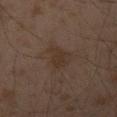The lesion was photographed on a routine skin check and not biopsied; there is no pathology result. The tile uses cross-polarized illumination. Cropped from a total-body skin-imaging series; the visible field is about 15 mm. A male subject, aged 58–62. The lesion-visualizer software estimated an area of roughly 4 mm², an eccentricity of roughly 0.8, and a symmetry-axis asymmetry near 0.4. And it measured a mean CIELAB color near L≈28 a*≈12 b*≈21, about 5 CIELAB-L* units darker than the surrounding skin, and a normalized lesion–skin contrast near 6. The analysis additionally found an automated nevus-likeness rating near 10 out of 100. From the right thigh. Longest diameter approximately 3 mm.